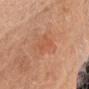Assessment:
The lesion was tiled from a total-body skin photograph and was not biopsied.
Acquisition and patient details:
Automated tile analysis of the lesion measured a shape eccentricity near 0.75 and two-axis asymmetry of about 0.2. And it measured a border-irregularity rating of about 2.5/10, internal color variation of about 3.5 on a 0–10 scale, and radial color variation of about 1. And it measured lesion-presence confidence of about 100/100. From the head or neck. Cropped from a whole-body photographic skin survey; the tile spans about 15 mm. Imaged with white-light lighting. A female patient, roughly 70 years of age. The recorded lesion diameter is about 3.5 mm.